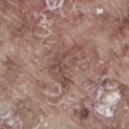Imaged during a routine full-body skin examination; the lesion was not biopsied and no histopathology is available.
On the right thigh.
Automated tile analysis of the lesion measured an average lesion color of about L≈48 a*≈18 b*≈23 (CIELAB), about 8 CIELAB-L* units darker than the surrounding skin, and a lesion-to-skin contrast of about 6 (normalized; higher = more distinct). The analysis additionally found a border-irregularity rating of about 10/10, a color-variation rating of about 2/10, and peripheral color asymmetry of about 0.5.
Captured under white-light illumination.
Cropped from a total-body skin-imaging series; the visible field is about 15 mm.
A male subject, in their mid-70s.